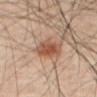| feature | finding |
|---|---|
| workup | catalogued during a skin exam; not biopsied |
| acquisition | ~15 mm crop, total-body skin-cancer survey |
| patient | male, aged around 55 |
| location | the abdomen |
| image-analysis metrics | a lesion color around L≈53 a*≈22 b*≈31 in CIELAB and a normalized lesion–skin contrast near 8.5; an automated nevus-likeness rating near 95 out of 100 and a lesion-detection confidence of about 100/100 |
| tile lighting | cross-polarized |